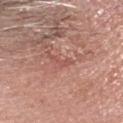Clinical impression:
The lesion was photographed on a routine skin check and not biopsied; there is no pathology result.
Image and clinical context:
Imaged with white-light lighting. A male subject in their mid- to late 60s. The lesion is located on the head or neck. Cropped from a whole-body photographic skin survey; the tile spans about 15 mm. Measured at roughly 2.5 mm in maximum diameter.